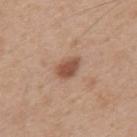Q: What kind of image is this?
A: total-body-photography crop, ~15 mm field of view
Q: Illumination type?
A: white-light illumination
Q: What is the anatomic site?
A: the upper back
Q: What is the lesion's diameter?
A: about 3 mm
Q: Patient demographics?
A: male, aged approximately 30